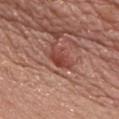Impression:
The lesion was tiled from a total-body skin photograph and was not biopsied.
Clinical summary:
On the front of the torso. The subject is a male in their mid-70s. Cropped from a total-body skin-imaging series; the visible field is about 15 mm.